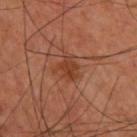Q: Was this lesion biopsied?
A: imaged on a skin check; not biopsied
Q: How was this image acquired?
A: total-body-photography crop, ~15 mm field of view
Q: What are the patient's age and sex?
A: male, aged 58–62
Q: What is the anatomic site?
A: the upper back